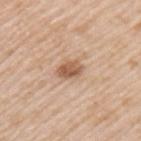Q: Is there a histopathology result?
A: total-body-photography surveillance lesion; no biopsy
Q: How was the tile lit?
A: white-light
Q: Automated lesion metrics?
A: a lesion area of about 4.5 mm²
Q: Who is the patient?
A: male, aged approximately 50
Q: Lesion location?
A: the upper back
Q: How large is the lesion?
A: about 3 mm
Q: How was this image acquired?
A: ~15 mm crop, total-body skin-cancer survey